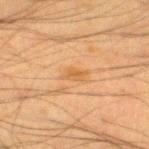Recorded during total-body skin imaging; not selected for excision or biopsy. Approximately 2.5 mm at its widest. A male patient roughly 35 years of age. Cropped from a whole-body photographic skin survey; the tile spans about 15 mm. An algorithmic analysis of the crop reported an average lesion color of about L≈48 a*≈18 b*≈35 (CIELAB) and a normalized border contrast of about 5.5. On the left thigh.